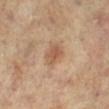<record>
  <biopsy_status>not biopsied; imaged during a skin examination</biopsy_status>
  <lighting>cross-polarized</lighting>
  <patient>
    <sex>female</sex>
    <age_approx>65</age_approx>
  </patient>
  <site>left leg</site>
  <image>
    <source>total-body photography crop</source>
    <field_of_view_mm>15</field_of_view_mm>
  </image>
  <automated_metrics>
    <area_mm2_approx>4.5</area_mm2_approx>
    <eccentricity>0.6</eccentricity>
    <shape_asymmetry>0.2</shape_asymmetry>
    <nevus_likeness_0_100>70</nevus_likeness_0_100>
    <lesion_detection_confidence_0_100>100</lesion_detection_confidence_0_100>
  </automated_metrics>
</record>